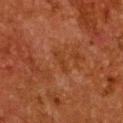No biopsy was performed on this lesion — it was imaged during a full skin examination and was not determined to be concerning.
Cropped from a whole-body photographic skin survey; the tile spans about 15 mm.
The lesion is on the chest.
The subject is a female aged around 50.